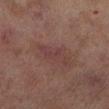* diameter · ~4.5 mm (longest diameter)
* automated lesion analysis · a lesion color around L≈34 a*≈18 b*≈17 in CIELAB, a lesion–skin lightness drop of about 5, and a normalized border contrast of about 5
* imaging modality · 15 mm crop, total-body photography
* lighting · cross-polarized illumination
* subject · female, aged 58 to 62
* anatomic site · the leg